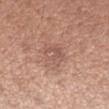Recorded during total-body skin imaging; not selected for excision or biopsy.
On the arm.
The tile uses white-light illumination.
A roughly 15 mm field-of-view crop from a total-body skin photograph.
A female patient, aged 28 to 32.